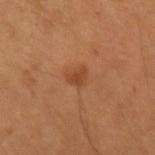Findings:
– biopsy status: no biopsy performed (imaged during a skin exam)
– patient: female, aged 43 to 47
– TBP lesion metrics: a border-irregularity rating of about 3/10, internal color variation of about 1 on a 0–10 scale, and peripheral color asymmetry of about 0.5; an automated nevus-likeness rating near 70 out of 100 and a detector confidence of about 100 out of 100 that the crop contains a lesion
– size: ~2.5 mm (longest diameter)
– tile lighting: cross-polarized
– image source: ~15 mm tile from a whole-body skin photo
– location: the right forearm